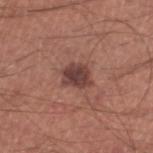The lesion was photographed on a routine skin check and not biopsied; there is no pathology result. About 3.5 mm across. Automated tile analysis of the lesion measured a peripheral color-asymmetry measure near 1. The software also gave a lesion-detection confidence of about 100/100. Captured under white-light illumination. Cropped from a total-body skin-imaging series; the visible field is about 15 mm. On the leg. A male subject, in their mid-40s.